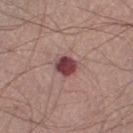notes: total-body-photography surveillance lesion; no biopsy.